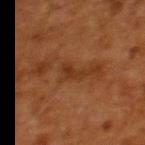{
  "biopsy_status": "not biopsied; imaged during a skin examination",
  "site": "upper back",
  "image": {
    "source": "total-body photography crop",
    "field_of_view_mm": 15
  },
  "patient": {
    "sex": "female",
    "age_approx": 50
  },
  "automated_metrics": {
    "shape_asymmetry": 0.55,
    "vs_skin_darker_L": 6.0,
    "vs_skin_contrast_norm": 7.0
  }
}